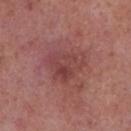diameter: about 5 mm
anatomic site: the right lower leg
image: total-body-photography crop, ~15 mm field of view
tile lighting: white-light
patient: female, about 40 years old
automated metrics: an eccentricity of roughly 0.6 and a symmetry-axis asymmetry near 0.4; internal color variation of about 5.5 on a 0–10 scale and radial color variation of about 2; lesion-presence confidence of about 100/100